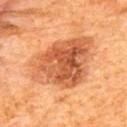biopsy status: catalogued during a skin exam; not biopsied
imaging modality: total-body-photography crop, ~15 mm field of view
body site: the upper back
tile lighting: cross-polarized
size: about 9 mm
subject: male, aged 63 to 67
TBP lesion metrics: a lesion area of about 34 mm² and a shape-asymmetry score of about 0.3 (0 = symmetric); a mean CIELAB color near L≈56 a*≈28 b*≈40, roughly 13 lightness units darker than nearby skin, and a lesion-to-skin contrast of about 8.5 (normalized; higher = more distinct); a classifier nevus-likeness of about 90/100